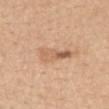  biopsy_status: not biopsied; imaged during a skin examination
  lighting: white-light
  site: mid back
  patient:
    sex: female
    age_approx: 65
  automated_metrics:
    cielab_L: 61
    cielab_a: 20
    cielab_b: 34
    vs_skin_darker_L: 10.0
    vs_skin_contrast_norm: 6.5
    border_irregularity_0_10: 5.0
    color_variation_0_10: 5.0
    peripheral_color_asymmetry: 2.0
    nevus_likeness_0_100: 5
  lesion_size:
    long_diameter_mm_approx: 5.0
  image:
    source: total-body photography crop
    field_of_view_mm: 15